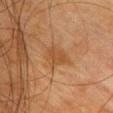Q: Was a biopsy performed?
A: total-body-photography surveillance lesion; no biopsy
Q: Lesion size?
A: ~3 mm (longest diameter)
Q: Automated lesion metrics?
A: an average lesion color of about L≈44 a*≈20 b*≈35 (CIELAB) and roughly 6 lightness units darker than nearby skin; a border-irregularity index near 3.5/10, internal color variation of about 2 on a 0–10 scale, and a peripheral color-asymmetry measure near 1
Q: What lighting was used for the tile?
A: cross-polarized
Q: What are the patient's age and sex?
A: female, aged approximately 55
Q: What is the anatomic site?
A: the chest
Q: What kind of image is this?
A: ~15 mm tile from a whole-body skin photo